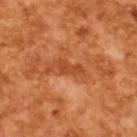The lesion was photographed on a routine skin check and not biopsied; there is no pathology result. The total-body-photography lesion software estimated a footprint of about 4 mm² and an outline eccentricity of about 0.9 (0 = round, 1 = elongated). About 3.5 mm across. A male subject, aged 63–67. A 15 mm close-up extracted from a 3D total-body photography capture.